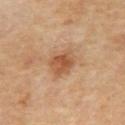follow-up: no biopsy performed (imaged during a skin exam); lesion diameter: ≈4 mm; acquisition: total-body-photography crop, ~15 mm field of view; subject: male, aged approximately 65; site: the mid back.